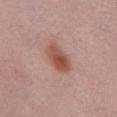The lesion was photographed on a routine skin check and not biopsied; there is no pathology result. A 15 mm close-up tile from a total-body photography series done for melanoma screening. Imaged with white-light lighting. A female patient roughly 65 years of age. On the abdomen. About 4.5 mm across.